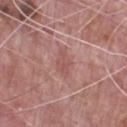The lesion is located on the chest. About 3.5 mm across. A 15 mm close-up extracted from a 3D total-body photography capture. A male patient, aged 68 to 72.